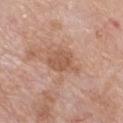Part of a total-body skin-imaging series; this lesion was reviewed on a skin check and was not flagged for biopsy. The subject is a female about 70 years old. Cropped from a total-body skin-imaging series; the visible field is about 15 mm. Longest diameter approximately 4.5 mm. The lesion is on the front of the torso.